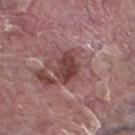biopsy status — total-body-photography surveillance lesion; no biopsy
automated metrics — an eccentricity of roughly 0.75 and two-axis asymmetry of about 0.2; a mean CIELAB color near L≈42 a*≈23 b*≈20 and about 11 CIELAB-L* units darker than the surrounding skin
anatomic site — the left lower leg
subject — male, approximately 40 years of age
tile lighting — white-light illumination
imaging modality — ~15 mm crop, total-body skin-cancer survey
size — ~4 mm (longest diameter)